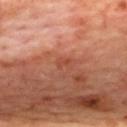site = the upper back
lighting = cross-polarized
patient = female, aged 43–47
diameter = ≈1.5 mm
imaging modality = ~15 mm tile from a whole-body skin photo